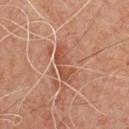Imaged with cross-polarized lighting. Located on the chest. The recorded lesion diameter is about 5 mm. A 15 mm close-up tile from a total-body photography series done for melanoma screening. A male subject approximately 60 years of age.